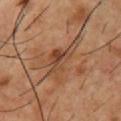workup: total-body-photography surveillance lesion; no biopsy
TBP lesion metrics: a footprint of about 11 mm² and a shape-asymmetry score of about 0.5 (0 = symmetric); an average lesion color of about L≈44 a*≈20 b*≈31 (CIELAB) and about 9 CIELAB-L* units darker than the surrounding skin; an automated nevus-likeness rating near 5 out of 100
subject: male, aged 53–57
lesion size: about 6 mm
lighting: cross-polarized illumination
imaging modality: total-body-photography crop, ~15 mm field of view
anatomic site: the chest